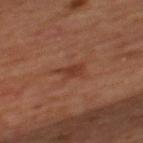Clinical impression: No biopsy was performed on this lesion — it was imaged during a full skin examination and was not determined to be concerning. Context: The lesion is on the mid back. Cropped from a total-body skin-imaging series; the visible field is about 15 mm. This is a cross-polarized tile. A male patient aged around 65. An algorithmic analysis of the crop reported a footprint of about 4 mm², an eccentricity of roughly 0.85, and a shape-asymmetry score of about 0.45 (0 = symmetric). The analysis additionally found a lesion color around L≈33 a*≈21 b*≈27 in CIELAB, about 7 CIELAB-L* units darker than the surrounding skin, and a lesion-to-skin contrast of about 6.5 (normalized; higher = more distinct).